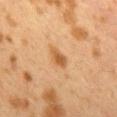Captured during whole-body skin photography for melanoma surveillance; the lesion was not biopsied.
The patient is a female in their 40s.
Located on the back.
The tile uses cross-polarized illumination.
A 15 mm close-up extracted from a 3D total-body photography capture.
An algorithmic analysis of the crop reported a lesion area of about 3.5 mm² and a shape-asymmetry score of about 0.3 (0 = symmetric). And it measured a border-irregularity rating of about 2.5/10 and peripheral color asymmetry of about 0.5.